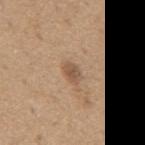Recorded during total-body skin imaging; not selected for excision or biopsy.